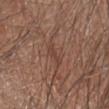Longest diameter approximately 2.5 mm. The patient is a male about 55 years old. The lesion is on the left forearm. A lesion tile, about 15 mm wide, cut from a 3D total-body photograph. Automated tile analysis of the lesion measured lesion-presence confidence of about 90/100. The tile uses white-light illumination.